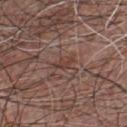The lesion was photographed on a routine skin check and not biopsied; there is no pathology result. A male patient, roughly 50 years of age. This image is a 15 mm lesion crop taken from a total-body photograph. This is a white-light tile. Located on the chest.